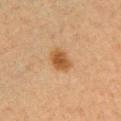Q: Was this lesion biopsied?
A: no biopsy performed (imaged during a skin exam)
Q: Patient demographics?
A: female, aged 38–42
Q: Where on the body is the lesion?
A: the front of the torso
Q: How was this image acquired?
A: ~15 mm tile from a whole-body skin photo